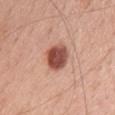| key | value |
|---|---|
| biopsy status | imaged on a skin check; not biopsied |
| site | the upper back |
| lighting | white-light illumination |
| patient | female, in their mid-40s |
| lesion diameter | about 3.5 mm |
| image | ~15 mm tile from a whole-body skin photo |